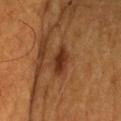Recorded during total-body skin imaging; not selected for excision or biopsy.
A close-up tile cropped from a whole-body skin photograph, about 15 mm across.
The lesion is on the head or neck.
A male patient in their mid- to late 70s.